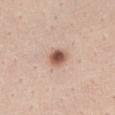biopsy_status: not biopsied; imaged during a skin examination
image:
  source: total-body photography crop
  field_of_view_mm: 15
lesion_size:
  long_diameter_mm_approx: 3.0
automated_metrics:
  eccentricity: 0.55
  cielab_L: 58
  cielab_a: 20
  cielab_b: 27
  vs_skin_darker_L: 15.0
  vs_skin_contrast_norm: 9.5
  border_irregularity_0_10: 2.0
  peripheral_color_asymmetry: 2.0
  nevus_likeness_0_100: 95
lighting: white-light
site: chest
patient:
  sex: female
  age_approx: 35